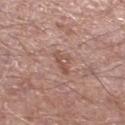Imaged during a routine full-body skin examination; the lesion was not biopsied and no histopathology is available.
A male patient aged 53 to 57.
The lesion is on the left lower leg.
Imaged with white-light lighting.
A 15 mm crop from a total-body photograph taken for skin-cancer surveillance.
Longest diameter approximately 3 mm.